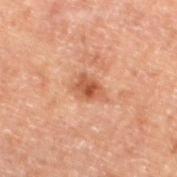The lesion was photographed on a routine skin check and not biopsied; there is no pathology result. A male patient, roughly 70 years of age. Measured at roughly 3 mm in maximum diameter. Automated tile analysis of the lesion measured a lesion area of about 5.5 mm², a shape eccentricity near 0.65, and a shape-asymmetry score of about 0.3 (0 = symmetric). The software also gave a lesion color around L≈42 a*≈21 b*≈28 in CIELAB, a lesion–skin lightness drop of about 9, and a normalized border contrast of about 7.5. The software also gave a border-irregularity rating of about 3/10, a color-variation rating of about 4/10, and peripheral color asymmetry of about 1. A roughly 15 mm field-of-view crop from a total-body skin photograph. From the left lower leg. Imaged with cross-polarized lighting.